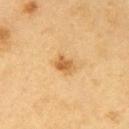follow-up = total-body-photography surveillance lesion; no biopsy | lighting = cross-polarized | subject = male, in their 60s | acquisition = ~15 mm tile from a whole-body skin photo | site = the arm | lesion size = ~2.5 mm (longest diameter).